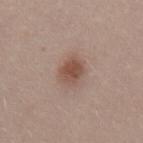biopsy status: total-body-photography surveillance lesion; no biopsy | image source: ~15 mm tile from a whole-body skin photo | patient: male, aged around 30 | body site: the mid back | tile lighting: white-light | TBP lesion metrics: an area of roughly 6 mm², a shape eccentricity near 0.5, and a shape-asymmetry score of about 0.15 (0 = symmetric); an automated nevus-likeness rating near 95 out of 100 | diameter: ≈3 mm.